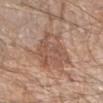Q: Was this lesion biopsied?
A: total-body-photography surveillance lesion; no biopsy
Q: How was the tile lit?
A: white-light illumination
Q: How was this image acquired?
A: ~15 mm tile from a whole-body skin photo
Q: Patient demographics?
A: male, about 70 years old
Q: Lesion location?
A: the right forearm